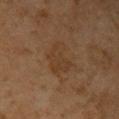biopsy_status: not biopsied; imaged during a skin examination
automated_metrics:
  area_mm2_approx: 10.0
  eccentricity: 0.6
  shape_asymmetry: 0.4
  lesion_detection_confidence_0_100: 100
lesion_size:
  long_diameter_mm_approx: 4.0
patient:
  sex: female
  age_approx: 60
site: left upper arm
image:
  source: total-body photography crop
  field_of_view_mm: 15